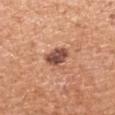The lesion was photographed on a routine skin check and not biopsied; there is no pathology result. The patient is a male aged 53–57. The tile uses white-light illumination. A 15 mm crop from a total-body photograph taken for skin-cancer surveillance. The lesion is on the left forearm. The recorded lesion diameter is about 3 mm.